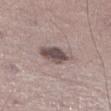Findings:
* workup — no biopsy performed (imaged during a skin exam)